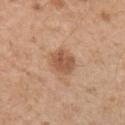Impression:
Captured during whole-body skin photography for melanoma surveillance; the lesion was not biopsied.
Acquisition and patient details:
The lesion-visualizer software estimated a lesion area of about 8 mm², a shape eccentricity near 0.55, and two-axis asymmetry of about 0.15. Located on the left upper arm. A female subject aged 38 to 42. A 15 mm close-up tile from a total-body photography series done for melanoma screening. The recorded lesion diameter is about 3.5 mm.